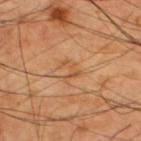Assessment:
No biopsy was performed on this lesion — it was imaged during a full skin examination and was not determined to be concerning.
Image and clinical context:
The subject is a male about 60 years old. Cropped from a total-body skin-imaging series; the visible field is about 15 mm. The lesion is located on the upper back.